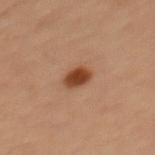The lesion was photographed on a routine skin check and not biopsied; there is no pathology result.
The lesion's longest dimension is about 3 mm.
On the mid back.
This is a cross-polarized tile.
A close-up tile cropped from a whole-body skin photograph, about 15 mm across.
A male patient, aged 33–37.